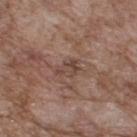{"biopsy_status": "not biopsied; imaged during a skin examination", "patient": {"sex": "male", "age_approx": 70}, "image": {"source": "total-body photography crop", "field_of_view_mm": 15}, "site": "upper back", "lighting": "white-light", "lesion_size": {"long_diameter_mm_approx": 3.5}}